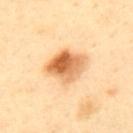anatomic site: the upper back; lighting: cross-polarized; subject: male, aged 38–42; image: 15 mm crop, total-body photography.